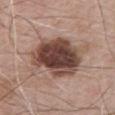Context: About 7 mm across. Automated image analysis of the tile measured a footprint of about 26 mm², an outline eccentricity of about 0.75 (0 = round, 1 = elongated), and a shape-asymmetry score of about 0.15 (0 = symmetric). The software also gave a classifier nevus-likeness of about 25/100. The lesion is located on the chest. The subject is a male approximately 65 years of age. A roughly 15 mm field-of-view crop from a total-body skin photograph.